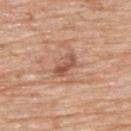{"biopsy_status": "not biopsied; imaged during a skin examination"}